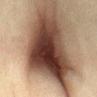<tbp_lesion>
  <biopsy_status>not biopsied; imaged during a skin examination</biopsy_status>
  <image>
    <source>total-body photography crop</source>
    <field_of_view_mm>15</field_of_view_mm>
  </image>
  <site>abdomen</site>
  <patient>
    <sex>female</sex>
    <age_approx>40</age_approx>
  </patient>
</tbp_lesion>